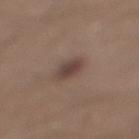{
  "biopsy_status": "not biopsied; imaged during a skin examination",
  "image": {
    "source": "total-body photography crop",
    "field_of_view_mm": 15
  },
  "patient": {
    "sex": "male",
    "age_approx": 30
  },
  "lighting": "white-light",
  "site": "leg",
  "lesion_size": {
    "long_diameter_mm_approx": 3.0
  },
  "automated_metrics": {
    "border_irregularity_0_10": 2.0,
    "color_variation_0_10": 3.5
  }
}